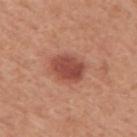Q: Was a biopsy performed?
A: no biopsy performed (imaged during a skin exam)
Q: Illumination type?
A: white-light
Q: How large is the lesion?
A: about 4.5 mm
Q: What kind of image is this?
A: 15 mm crop, total-body photography
Q: What are the patient's age and sex?
A: male, roughly 50 years of age
Q: Lesion location?
A: the arm